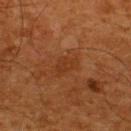Recorded during total-body skin imaging; not selected for excision or biopsy.